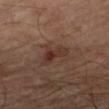A male subject about 65 years old.
A lesion tile, about 15 mm wide, cut from a 3D total-body photograph.
The lesion is on the right upper arm.